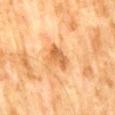{
  "biopsy_status": "not biopsied; imaged during a skin examination",
  "site": "front of the torso",
  "image": {
    "source": "total-body photography crop",
    "field_of_view_mm": 15
  },
  "patient": {
    "sex": "male",
    "age_approx": 60
  }
}